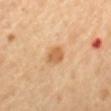Impression: The lesion was tiled from a total-body skin photograph and was not biopsied. Background: From the mid back. Cropped from a total-body skin-imaging series; the visible field is about 15 mm. The recorded lesion diameter is about 2.5 mm. A female patient, approximately 65 years of age.